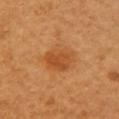body site: the right upper arm
lesion diameter: ~3.5 mm (longest diameter)
imaging modality: 15 mm crop, total-body photography
subject: female, approximately 55 years of age